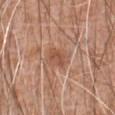Findings:
– notes · imaged on a skin check; not biopsied
– subject · male, roughly 60 years of age
– anatomic site · the back
– tile lighting · white-light
– size · ~2.5 mm (longest diameter)
– acquisition · total-body-photography crop, ~15 mm field of view